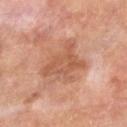Impression: No biopsy was performed on this lesion — it was imaged during a full skin examination and was not determined to be concerning. Image and clinical context: A region of skin cropped from a whole-body photographic capture, roughly 15 mm wide. The lesion is on the right lower leg. The patient is a male in their mid-60s.